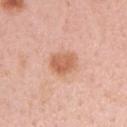No biopsy was performed on this lesion — it was imaged during a full skin examination and was not determined to be concerning.
This image is a 15 mm lesion crop taken from a total-body photograph.
The subject is a female in their mid- to late 30s.
From the left upper arm.